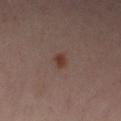biopsy status: no biopsy performed (imaged during a skin exam)
image source: ~15 mm tile from a whole-body skin photo
subject: female, roughly 40 years of age
body site: the left lower leg
image-analysis metrics: about 8 CIELAB-L* units darker than the surrounding skin and a normalized border contrast of about 8.5; a border-irregularity rating of about 1/10 and peripheral color asymmetry of about 0.5
lesion diameter: ≈2 mm
illumination: cross-polarized illumination